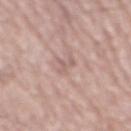Findings:
- subject: male, aged around 75
- acquisition: ~15 mm crop, total-body skin-cancer survey
- site: the mid back